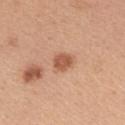Q: Is there a histopathology result?
A: no biopsy performed (imaged during a skin exam)
Q: How was the tile lit?
A: white-light illumination
Q: Automated lesion metrics?
A: a lesion area of about 5 mm² and an eccentricity of roughly 0.6; a border-irregularity index near 2/10 and peripheral color asymmetry of about 0.5
Q: How was this image acquired?
A: 15 mm crop, total-body photography
Q: Lesion size?
A: ≈2.5 mm
Q: What are the patient's age and sex?
A: female, in their mid-40s
Q: Lesion location?
A: the left upper arm